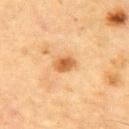Q: Was this lesion biopsied?
A: imaged on a skin check; not biopsied
Q: What did automated image analysis measure?
A: an area of roughly 4.5 mm², a shape eccentricity near 0.8, and two-axis asymmetry of about 0.2; a border-irregularity index near 2/10, a color-variation rating of about 4.5/10, and radial color variation of about 1.5
Q: Lesion location?
A: the front of the torso
Q: How was the tile lit?
A: cross-polarized
Q: Who is the patient?
A: male, aged 83 or over
Q: Lesion size?
A: ≈3 mm
Q: What kind of image is this?
A: total-body-photography crop, ~15 mm field of view